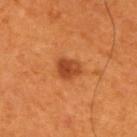| field | value |
|---|---|
| workup | no biopsy performed (imaged during a skin exam) |
| site | the upper back |
| acquisition | total-body-photography crop, ~15 mm field of view |
| patient | male, in their 60s |